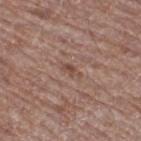Imaged during a routine full-body skin examination; the lesion was not biopsied and no histopathology is available. This is a white-light tile. Cropped from a whole-body photographic skin survey; the tile spans about 15 mm. A male subject, aged around 70. Measured at roughly 2.5 mm in maximum diameter. The total-body-photography lesion software estimated a color-variation rating of about 0/10 and a peripheral color-asymmetry measure near 0. It also reported lesion-presence confidence of about 95/100. On the right thigh.